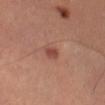Impression:
Recorded during total-body skin imaging; not selected for excision or biopsy.
Background:
This is a cross-polarized tile. A 15 mm close-up extracted from a 3D total-body photography capture. A female subject, aged approximately 45. An algorithmic analysis of the crop reported a lesion area of about 2 mm², an outline eccentricity of about 0.7 (0 = round, 1 = elongated), and two-axis asymmetry of about 0.25. The analysis additionally found an automated nevus-likeness rating near 85 out of 100. On the leg. The lesion's longest dimension is about 2 mm.